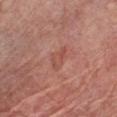Q: Was this lesion biopsied?
A: no biopsy performed (imaged during a skin exam)
Q: Patient demographics?
A: male, aged approximately 80
Q: How large is the lesion?
A: ~3 mm (longest diameter)
Q: What is the imaging modality?
A: ~15 mm tile from a whole-body skin photo
Q: What is the anatomic site?
A: the chest